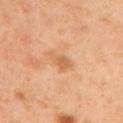The lesion was tiled from a total-body skin photograph and was not biopsied.